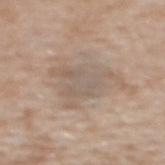biopsy status = imaged on a skin check; not biopsied
body site = the back
tile lighting = white-light
diameter = about 6 mm
subject = female, aged 43–47
image source = ~15 mm crop, total-body skin-cancer survey